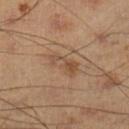Q: Was this lesion biopsied?
A: no biopsy performed (imaged during a skin exam)
Q: Who is the patient?
A: male, about 40 years old
Q: How was the tile lit?
A: cross-polarized illumination
Q: Where on the body is the lesion?
A: the left lower leg
Q: How was this image acquired?
A: ~15 mm tile from a whole-body skin photo